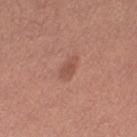Clinical impression: No biopsy was performed on this lesion — it was imaged during a full skin examination and was not determined to be concerning. Image and clinical context: Imaged with white-light lighting. A 15 mm crop from a total-body photograph taken for skin-cancer surveillance. Located on the left lower leg. The subject is a female approximately 50 years of age.